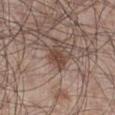Assessment:
Captured during whole-body skin photography for melanoma surveillance; the lesion was not biopsied.
Image and clinical context:
This image is a 15 mm lesion crop taken from a total-body photograph. Located on the right lower leg. A male subject roughly 55 years of age.